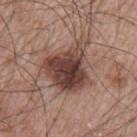<lesion>
<biopsy_status>not biopsied; imaged during a skin examination</biopsy_status>
<lighting>white-light</lighting>
<image>
  <source>total-body photography crop</source>
  <field_of_view_mm>15</field_of_view_mm>
</image>
<lesion_size>
  <long_diameter_mm_approx>7.0</long_diameter_mm_approx>
</lesion_size>
<patient>
  <sex>male</sex>
  <age_approx>65</age_approx>
</patient>
<site>left upper arm</site>
</lesion>